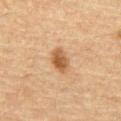biopsy_status: not biopsied; imaged during a skin examination
lesion_size:
  long_diameter_mm_approx: 3.0
site: abdomen
patient:
  sex: male
  age_approx: 65
image:
  source: total-body photography crop
  field_of_view_mm: 15
automated_metrics:
  area_mm2_approx: 5.0
  eccentricity: 0.8
  cielab_L: 46
  cielab_a: 18
  cielab_b: 33
  vs_skin_darker_L: 11.0
  vs_skin_contrast_norm: 9.0
  border_irregularity_0_10: 1.5
  color_variation_0_10: 3.5
  peripheral_color_asymmetry: 1.0
  nevus_likeness_0_100: 90
lighting: cross-polarized